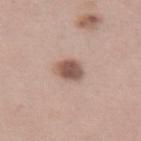Findings:
– workup: catalogued during a skin exam; not biopsied
– TBP lesion metrics: a lesion area of about 6 mm², a shape eccentricity near 0.6, and a shape-asymmetry score of about 0.15 (0 = symmetric); border irregularity of about 1.5 on a 0–10 scale and a within-lesion color-variation index near 4/10; a classifier nevus-likeness of about 90/100 and a lesion-detection confidence of about 100/100
– image: total-body-photography crop, ~15 mm field of view
– size: ≈3 mm
– subject: female, in their mid- to late 40s
– anatomic site: the left thigh